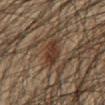Impression: Part of a total-body skin-imaging series; this lesion was reviewed on a skin check and was not flagged for biopsy. Clinical summary: A male patient, aged 58 to 62. A 15 mm close-up extracted from a 3D total-body photography capture. The lesion is located on the front of the torso.